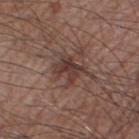Recorded during total-body skin imaging; not selected for excision or biopsy.
The lesion is located on the right thigh.
A male subject aged 58 to 62.
About 5 mm across.
Automated tile analysis of the lesion measured a lesion area of about 9.5 mm² and an outline eccentricity of about 0.7 (0 = round, 1 = elongated). It also reported a lesion–skin lightness drop of about 9 and a lesion-to-skin contrast of about 7.5 (normalized; higher = more distinct). The software also gave a border-irregularity rating of about 6/10 and radial color variation of about 2. The software also gave a classifier nevus-likeness of about 5/100 and a detector confidence of about 100 out of 100 that the crop contains a lesion.
A 15 mm close-up tile from a total-body photography series done for melanoma screening.